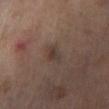{"biopsy_status": "not biopsied; imaged during a skin examination", "image": {"source": "total-body photography crop", "field_of_view_mm": 15}, "site": "left lower leg", "patient": {"sex": "male", "age_approx": 70}, "lesion_size": {"long_diameter_mm_approx": 2.5}}